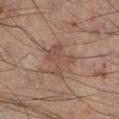biopsy status = imaged on a skin check; not biopsied | acquisition = ~15 mm crop, total-body skin-cancer survey | patient = male, roughly 60 years of age | size = about 4 mm | site = the left lower leg | tile lighting = cross-polarized illumination.